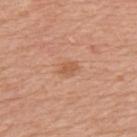Cropped from a total-body skin-imaging series; the visible field is about 15 mm. Located on the back. A female patient, aged 38 to 42. Captured under white-light illumination. The lesion-visualizer software estimated a lesion color around L≈58 a*≈24 b*≈35 in CIELAB, about 8 CIELAB-L* units darker than the surrounding skin, and a lesion-to-skin contrast of about 6 (normalized; higher = more distinct). And it measured border irregularity of about 2.5 on a 0–10 scale and peripheral color asymmetry of about 0.5.